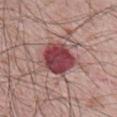| field | value |
|---|---|
| biopsy status | no biopsy performed (imaged during a skin exam) |
| patient | male, about 65 years old |
| TBP lesion metrics | a lesion area of about 14 mm², an eccentricity of roughly 0.75, and a symmetry-axis asymmetry near 0.15; a mean CIELAB color near L≈43 a*≈30 b*≈19, a lesion–skin lightness drop of about 17, and a lesion-to-skin contrast of about 12.5 (normalized; higher = more distinct); border irregularity of about 1.5 on a 0–10 scale, a within-lesion color-variation index near 5.5/10, and radial color variation of about 2; a nevus-likeness score of about 60/100 and lesion-presence confidence of about 100/100 |
| tile lighting | white-light |
| imaging modality | total-body-photography crop, ~15 mm field of view |
| lesion size | ~5.5 mm (longest diameter) |
| location | the upper back |